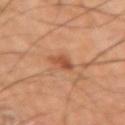The lesion was tiled from a total-body skin photograph and was not biopsied. The lesion-visualizer software estimated a footprint of about 4 mm² and an eccentricity of roughly 0.8. A male subject approximately 60 years of age. A region of skin cropped from a whole-body photographic capture, roughly 15 mm wide. From the right upper arm.